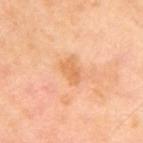Part of a total-body skin-imaging series; this lesion was reviewed on a skin check and was not flagged for biopsy. A female subject aged approximately 55. This image is a 15 mm lesion crop taken from a total-body photograph. Longest diameter approximately 3 mm. On the right upper arm.